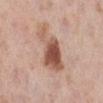No biopsy was performed on this lesion — it was imaged during a full skin examination and was not determined to be concerning. Imaged with white-light lighting. Located on the right lower leg. The patient is a female approximately 65 years of age. A 15 mm close-up extracted from a 3D total-body photography capture. Automated tile analysis of the lesion measured an outline eccentricity of about 0.85 (0 = round, 1 = elongated) and a shape-asymmetry score of about 0.4 (0 = symmetric). It also reported a border-irregularity rating of about 5.5/10 and internal color variation of about 6 on a 0–10 scale. The analysis additionally found a nevus-likeness score of about 75/100 and a detector confidence of about 100 out of 100 that the crop contains a lesion.